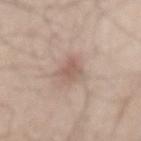No biopsy was performed on this lesion — it was imaged during a full skin examination and was not determined to be concerning.
A male patient, aged 43–47.
The lesion's longest dimension is about 3 mm.
The tile uses white-light illumination.
A close-up tile cropped from a whole-body skin photograph, about 15 mm across.
Located on the lower back.